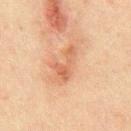Impression: Captured during whole-body skin photography for melanoma surveillance; the lesion was not biopsied. Image and clinical context: Imaged with cross-polarized lighting. Located on the abdomen. The patient is a male about 50 years old. Cropped from a total-body skin-imaging series; the visible field is about 15 mm.